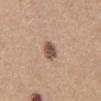Findings:
- biopsy status: no biopsy performed (imaged during a skin exam)
- location: the front of the torso
- image: ~15 mm crop, total-body skin-cancer survey
- subject: male, about 65 years old